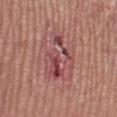The lesion was tiled from a total-body skin photograph and was not biopsied. The lesion is located on the right lower leg. Cropped from a whole-body photographic skin survey; the tile spans about 15 mm. A male subject aged 53–57.